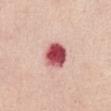Image and clinical context:
Imaged with white-light lighting. The total-body-photography lesion software estimated an area of roughly 8.5 mm², a shape eccentricity near 0.35, and a shape-asymmetry score of about 0.15 (0 = symmetric). The analysis additionally found roughly 23 lightness units darker than nearby skin and a normalized lesion–skin contrast near 14. The analysis additionally found a classifier nevus-likeness of about 0/100. The recorded lesion diameter is about 3.5 mm. A 15 mm close-up extracted from a 3D total-body photography capture. A female patient, approximately 55 years of age. On the chest.